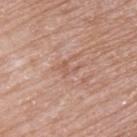| feature | finding |
|---|---|
| workup | no biopsy performed (imaged during a skin exam) |
| site | the back |
| image source | 15 mm crop, total-body photography |
| diameter | ~2.5 mm (longest diameter) |
| patient | male, in their mid-70s |
| lighting | white-light |